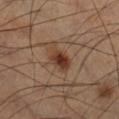{"biopsy_status": "not biopsied; imaged during a skin examination"}